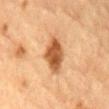Notes:
– workup — catalogued during a skin exam; not biopsied
– patient — male, aged around 85 or older
– location — the mid back
– automated metrics — a lesion color around L≈49 a*≈22 b*≈36 in CIELAB, a lesion–skin lightness drop of about 14, and a normalized lesion–skin contrast near 10
– tile lighting — cross-polarized illumination
– image — 15 mm crop, total-body photography
– lesion diameter — ~4 mm (longest diameter)